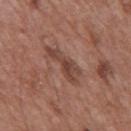No biopsy was performed on this lesion — it was imaged during a full skin examination and was not determined to be concerning. The tile uses white-light illumination. A male subject about 50 years old. Cropped from a total-body skin-imaging series; the visible field is about 15 mm. The lesion is on the mid back. Automated tile analysis of the lesion measured a mean CIELAB color near L≈43 a*≈21 b*≈26, a lesion–skin lightness drop of about 9, and a lesion-to-skin contrast of about 7 (normalized; higher = more distinct). And it measured a border-irregularity index near 6/10 and a peripheral color-asymmetry measure near 1. The software also gave an automated nevus-likeness rating near 0 out of 100 and a lesion-detection confidence of about 95/100.